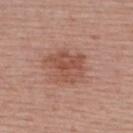Clinical impression: The lesion was tiled from a total-body skin photograph and was not biopsied. Image and clinical context: A female subject, about 55 years old. Located on the upper back. The total-body-photography lesion software estimated a footprint of about 12 mm², an outline eccentricity of about 0.55 (0 = round, 1 = elongated), and a shape-asymmetry score of about 0.3 (0 = symmetric). The analysis additionally found a classifier nevus-likeness of about 5/100. The recorded lesion diameter is about 4.5 mm. This image is a 15 mm lesion crop taken from a total-body photograph. Imaged with white-light lighting.